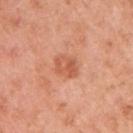Clinical impression: Recorded during total-body skin imaging; not selected for excision or biopsy. Context: The tile uses white-light illumination. An algorithmic analysis of the crop reported a footprint of about 5.5 mm², an eccentricity of roughly 0.7, and two-axis asymmetry of about 0.25. The software also gave a lesion color around L≈58 a*≈29 b*≈36 in CIELAB and a lesion–skin lightness drop of about 9. It also reported border irregularity of about 2.5 on a 0–10 scale, a color-variation rating of about 4.5/10, and radial color variation of about 1.5. The analysis additionally found an automated nevus-likeness rating near 5 out of 100 and lesion-presence confidence of about 100/100. The patient is a female aged around 50. From the right upper arm. A 15 mm close-up tile from a total-body photography series done for melanoma screening.